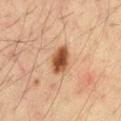Notes:
* biopsy status — imaged on a skin check; not biopsied
* subject — male, aged 33 to 37
* image source — 15 mm crop, total-body photography
* location — the chest